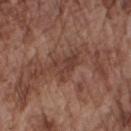Q: Is there a histopathology result?
A: imaged on a skin check; not biopsied
Q: Automated lesion metrics?
A: a symmetry-axis asymmetry near 0.45
Q: Lesion location?
A: the right upper arm
Q: Lesion size?
A: ~4 mm (longest diameter)
Q: Patient demographics?
A: male, aged 73–77
Q: What kind of image is this?
A: 15 mm crop, total-body photography